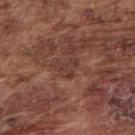Assessment:
Recorded during total-body skin imaging; not selected for excision or biopsy.